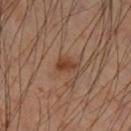{
  "biopsy_status": "not biopsied; imaged during a skin examination",
  "site": "left lower leg",
  "patient": {
    "sex": "male",
    "age_approx": 50
  },
  "image": {
    "source": "total-body photography crop",
    "field_of_view_mm": 15
  },
  "lighting": "cross-polarized",
  "automated_metrics": {
    "eccentricity": 0.8,
    "shape_asymmetry": 0.35,
    "cielab_L": 39,
    "cielab_a": 22,
    "cielab_b": 32,
    "vs_skin_contrast_norm": 9.0,
    "border_irregularity_0_10": 3.0,
    "peripheral_color_asymmetry": 0.5,
    "nevus_likeness_0_100": 75,
    "lesion_detection_confidence_0_100": 100
  }
}